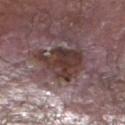Assessment:
The lesion was photographed on a routine skin check and not biopsied; there is no pathology result.
Background:
The lesion is on the left lower leg. This is a white-light tile. The patient is a male approximately 80 years of age. Automated image analysis of the tile measured a lesion color around L≈36 a*≈17 b*≈19 in CIELAB and a normalized border contrast of about 11.5. And it measured peripheral color asymmetry of about 1.5. Cropped from a total-body skin-imaging series; the visible field is about 15 mm. Approximately 6 mm at its widest.